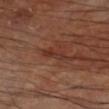Case summary:
* acquisition · ~15 mm tile from a whole-body skin photo
* subject · male, approximately 60 years of age
* tile lighting · cross-polarized
* site · the right lower leg
* diameter · ~3 mm (longest diameter)